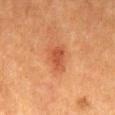From the mid back. Automated tile analysis of the lesion measured a lesion area of about 7.5 mm², an outline eccentricity of about 0.65 (0 = round, 1 = elongated), and a symmetry-axis asymmetry near 0.35. The software also gave a lesion color around L≈43 a*≈25 b*≈32 in CIELAB and a normalized border contrast of about 6. The software also gave a border-irregularity rating of about 3/10 and radial color variation of about 0.5. A male subject aged 48–52. Cropped from a total-body skin-imaging series; the visible field is about 15 mm. The tile uses cross-polarized illumination. Longest diameter approximately 3.5 mm.